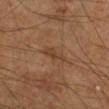This lesion was catalogued during total-body skin photography and was not selected for biopsy.